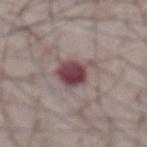The lesion was photographed on a routine skin check and not biopsied; there is no pathology result.
The recorded lesion diameter is about 4 mm.
Captured under white-light illumination.
Located on the abdomen.
A region of skin cropped from a whole-body photographic capture, roughly 15 mm wide.
A male subject, aged 73–77.